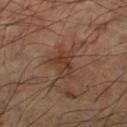workup: no biopsy performed (imaged during a skin exam) | automated metrics: a lesion area of about 7.5 mm² and a symmetry-axis asymmetry near 0.45; a mean CIELAB color near L≈36 a*≈19 b*≈26, about 7 CIELAB-L* units darker than the surrounding skin, and a normalized border contrast of about 6.5 | subject: male, roughly 60 years of age | anatomic site: the right thigh | acquisition: total-body-photography crop, ~15 mm field of view.